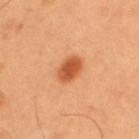Impression:
Captured during whole-body skin photography for melanoma surveillance; the lesion was not biopsied.
Image and clinical context:
On the upper back. A close-up tile cropped from a whole-body skin photograph, about 15 mm across. Measured at roughly 3 mm in maximum diameter. The patient is a male in their mid-50s.